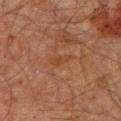Impression:
Captured during whole-body skin photography for melanoma surveillance; the lesion was not biopsied.
Background:
Located on the chest. A male patient aged approximately 60. A 15 mm close-up tile from a total-body photography series done for melanoma screening. The lesion-visualizer software estimated an average lesion color of about L≈33 a*≈18 b*≈27 (CIELAB), a lesion–skin lightness drop of about 4, and a normalized border contrast of about 5. It also reported border irregularity of about 3.5 on a 0–10 scale, a within-lesion color-variation index near 0/10, and radial color variation of about 0. This is a cross-polarized tile.